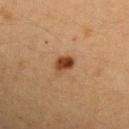{
  "biopsy_status": "not biopsied; imaged during a skin examination",
  "automated_metrics": {
    "area_mm2_approx": 4.0,
    "eccentricity": 0.75,
    "shape_asymmetry": 0.25,
    "cielab_L": 37,
    "cielab_a": 21,
    "cielab_b": 32,
    "vs_skin_darker_L": 13.0,
    "vs_skin_contrast_norm": 11.0,
    "color_variation_0_10": 4.0,
    "peripheral_color_asymmetry": 1.5
  },
  "patient": {
    "sex": "female",
    "age_approx": 40
  },
  "lighting": "cross-polarized",
  "site": "left upper arm",
  "lesion_size": {
    "long_diameter_mm_approx": 3.0
  },
  "image": {
    "source": "total-body photography crop",
    "field_of_view_mm": 15
  }
}